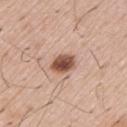  biopsy_status: not biopsied; imaged during a skin examination
  lighting: white-light
  patient:
    sex: male
    age_approx: 55
  image:
    source: total-body photography crop
    field_of_view_mm: 15